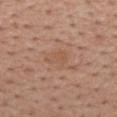Recorded during total-body skin imaging; not selected for excision or biopsy.